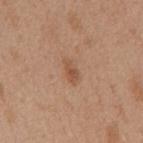The lesion was photographed on a routine skin check and not biopsied; there is no pathology result. The lesion is located on the back. The recorded lesion diameter is about 3.5 mm. This image is a 15 mm lesion crop taken from a total-body photograph. A female patient aged around 40. This is a white-light tile.